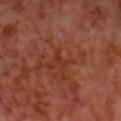Imaged during a routine full-body skin examination; the lesion was not biopsied and no histopathology is available.
Imaged with cross-polarized lighting.
A male subject, in their mid- to late 50s.
The lesion is on the head or neck.
Automated tile analysis of the lesion measured a mean CIELAB color near L≈35 a*≈28 b*≈31, about 5 CIELAB-L* units darker than the surrounding skin, and a normalized lesion–skin contrast near 5. The software also gave an automated nevus-likeness rating near 0 out of 100 and a detector confidence of about 95 out of 100 that the crop contains a lesion.
Cropped from a whole-body photographic skin survey; the tile spans about 15 mm.